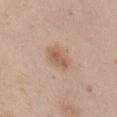– workup: no biopsy performed (imaged during a skin exam)
– acquisition: ~15 mm crop, total-body skin-cancer survey
– anatomic site: the right upper arm
– patient: male, roughly 45 years of age